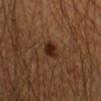A male patient, aged 63 to 67. The lesion's longest dimension is about 2.5 mm. From the right upper arm. The tile uses cross-polarized illumination. A 15 mm close-up tile from a total-body photography series done for melanoma screening.